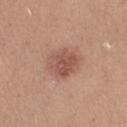Imaged during a routine full-body skin examination; the lesion was not biopsied and no histopathology is available. Captured under white-light illumination. The patient is a female about 30 years old. Longest diameter approximately 4 mm. The lesion is on the right thigh. A lesion tile, about 15 mm wide, cut from a 3D total-body photograph.